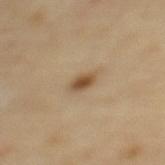- workup · total-body-photography surveillance lesion; no biopsy
- patient · female, approximately 65 years of age
- image · 15 mm crop, total-body photography
- illumination · cross-polarized illumination
- lesion size · ~2.5 mm (longest diameter)
- automated lesion analysis · border irregularity of about 2.5 on a 0–10 scale, a color-variation rating of about 3.5/10, and a peripheral color-asymmetry measure near 1; a classifier nevus-likeness of about 95/100
- body site · the upper back